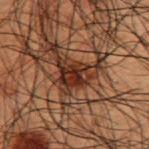workup: catalogued during a skin exam; not biopsied | patient: male, in their 50s | imaging modality: ~15 mm crop, total-body skin-cancer survey | location: the upper back | diameter: ≈5 mm.